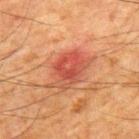This image is a 15 mm lesion crop taken from a total-body photograph.
The patient is a male aged approximately 65.
Approximately 5 mm at its widest.
On the upper back.
Imaged with cross-polarized lighting.